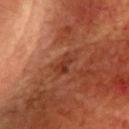  biopsy_status: not biopsied; imaged during a skin examination
  lesion_size:
    long_diameter_mm_approx: 3.0
  patient:
    sex: female
    age_approx: 80
  lighting: cross-polarized
  image:
    source: total-body photography crop
    field_of_view_mm: 15
  site: head or neck